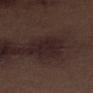Assessment: No biopsy was performed on this lesion — it was imaged during a full skin examination and was not determined to be concerning. Background: This is a white-light tile. A male patient about 70 years old. The recorded lesion diameter is about 3 mm. A 15 mm close-up tile from a total-body photography series done for melanoma screening. An algorithmic analysis of the crop reported a symmetry-axis asymmetry near 0.35. Located on the leg.